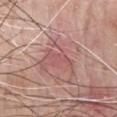<tbp_lesion>
  <biopsy_status>not biopsied; imaged during a skin examination</biopsy_status>
  <image>
    <source>total-body photography crop</source>
    <field_of_view_mm>15</field_of_view_mm>
  </image>
  <lesion_size>
    <long_diameter_mm_approx>4.0</long_diameter_mm_approx>
  </lesion_size>
  <automated_metrics>
    <area_mm2_approx>4.0</area_mm2_approx>
    <shape_asymmetry>0.5</shape_asymmetry>
    <cielab_L>54</cielab_L>
    <cielab_a>26</cielab_a>
    <cielab_b>21</cielab_b>
    <vs_skin_darker_L>7.0</vs_skin_darker_L>
    <lesion_detection_confidence_0_100>60</lesion_detection_confidence_0_100>
  </automated_metrics>
  <patient>
    <sex>male</sex>
    <age_approx>75</age_approx>
  </patient>
  <site>chest</site>
  <lighting>white-light</lighting>
</tbp_lesion>